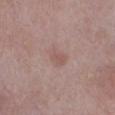Captured during whole-body skin photography for melanoma surveillance; the lesion was not biopsied. Longest diameter approximately 3 mm. Cropped from a total-body skin-imaging series; the visible field is about 15 mm. The patient is a female about 60 years old. This is a white-light tile. The lesion is located on the leg.